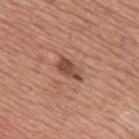workup = total-body-photography surveillance lesion; no biopsy | image source = ~15 mm crop, total-body skin-cancer survey | image-analysis metrics = a lesion area of about 5 mm² and two-axis asymmetry of about 0.35; a lesion-detection confidence of about 100/100 | body site = the upper back | illumination = white-light | subject = male, aged approximately 75 | lesion diameter = ~3.5 mm (longest diameter).